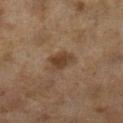Impression:
The lesion was photographed on a routine skin check and not biopsied; there is no pathology result.
Clinical summary:
The patient is a female aged 58–62. The lesion is located on the left lower leg. The tile uses cross-polarized illumination. This image is a 15 mm lesion crop taken from a total-body photograph.